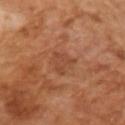<case>
  <biopsy_status>not biopsied; imaged during a skin examination</biopsy_status>
  <patient>
    <sex>male</sex>
    <age_approx>65</age_approx>
  </patient>
  <lesion_size>
    <long_diameter_mm_approx>3.0</long_diameter_mm_approx>
  </lesion_size>
  <automated_metrics>
    <eccentricity>0.7</eccentricity>
    <shape_asymmetry>0.45</shape_asymmetry>
    <color_variation_0_10>1.5</color_variation_0_10>
    <peripheral_color_asymmetry>0.5</peripheral_color_asymmetry>
    <nevus_likeness_0_100>0</nevus_likeness_0_100>
    <lesion_detection_confidence_0_100>100</lesion_detection_confidence_0_100>
  </automated_metrics>
  <image>
    <source>total-body photography crop</source>
    <field_of_view_mm>15</field_of_view_mm>
  </image>
</case>